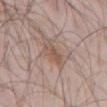- biopsy status: catalogued during a skin exam; not biopsied
- anatomic site: the abdomen
- automated metrics: a shape eccentricity near 0.85 and two-axis asymmetry of about 0.3; a classifier nevus-likeness of about 60/100 and a detector confidence of about 100 out of 100 that the crop contains a lesion
- image source: ~15 mm crop, total-body skin-cancer survey
- subject: male, aged 58–62
- tile lighting: white-light illumination